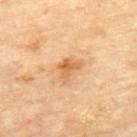notes: catalogued during a skin exam; not biopsied | image: 15 mm crop, total-body photography | subject: male, approximately 85 years of age or older | location: the upper back | size: ~2.5 mm (longest diameter) | tile lighting: cross-polarized.